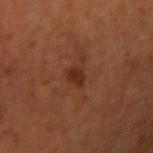Findings:
- site — the arm
- tile lighting — cross-polarized illumination
- size — ≈2.5 mm
- acquisition — ~15 mm tile from a whole-body skin photo
- patient — male, aged 58 to 62
- automated metrics — a footprint of about 3.5 mm², an outline eccentricity of about 0.7 (0 = round, 1 = elongated), and two-axis asymmetry of about 0.25; internal color variation of about 1.5 on a 0–10 scale and a peripheral color-asymmetry measure near 0.5